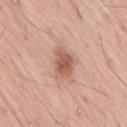The lesion was tiled from a total-body skin photograph and was not biopsied.
On the lower back.
The patient is a male in their mid- to late 50s.
A lesion tile, about 15 mm wide, cut from a 3D total-body photograph.